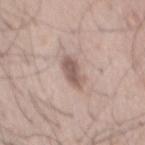This lesion was catalogued during total-body skin photography and was not selected for biopsy. This is a white-light tile. An algorithmic analysis of the crop reported a shape eccentricity near 0.9 and a symmetry-axis asymmetry near 0.3. And it measured a border-irregularity index near 3.5/10, internal color variation of about 2 on a 0–10 scale, and a peripheral color-asymmetry measure near 1. The software also gave a nevus-likeness score of about 50/100 and a detector confidence of about 75 out of 100 that the crop contains a lesion. A male subject aged 48–52. A roughly 15 mm field-of-view crop from a total-body skin photograph. The lesion is located on the back. About 4.5 mm across.